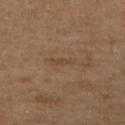This lesion was catalogued during total-body skin photography and was not selected for biopsy. Cropped from a whole-body photographic skin survey; the tile spans about 15 mm. The lesion is on the right lower leg. A male subject about 65 years old.